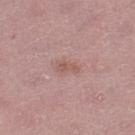Impression: No biopsy was performed on this lesion — it was imaged during a full skin examination and was not determined to be concerning. Image and clinical context: The patient is a female aged 43 to 47. Approximately 2.5 mm at its widest. Automated image analysis of the tile measured an area of roughly 2.5 mm², an eccentricity of roughly 0.9, and two-axis asymmetry of about 0.45. The software also gave a classifier nevus-likeness of about 0/100 and a lesion-detection confidence of about 100/100. Located on the leg. Cropped from a whole-body photographic skin survey; the tile spans about 15 mm.